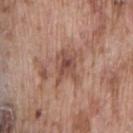Q: Was this lesion biopsied?
A: catalogued during a skin exam; not biopsied
Q: How was this image acquired?
A: total-body-photography crop, ~15 mm field of view
Q: What lighting was used for the tile?
A: white-light illumination
Q: Lesion size?
A: ~4 mm (longest diameter)
Q: Where on the body is the lesion?
A: the lower back
Q: What did automated image analysis measure?
A: a lesion area of about 7 mm², a shape eccentricity near 0.7, and two-axis asymmetry of about 0.4; a lesion color around L≈49 a*≈21 b*≈26 in CIELAB and a normalized border contrast of about 7; a color-variation rating of about 4.5/10 and a peripheral color-asymmetry measure near 1.5
Q: Patient demographics?
A: male, aged approximately 75